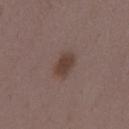Assessment:
No biopsy was performed on this lesion — it was imaged during a full skin examination and was not determined to be concerning.
Acquisition and patient details:
A female subject in their mid-30s. Cropped from a whole-body photographic skin survey; the tile spans about 15 mm. On the mid back.